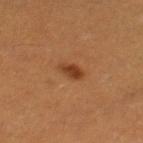<tbp_lesion>
<biopsy_status>not biopsied; imaged during a skin examination</biopsy_status>
<lesion_size>
  <long_diameter_mm_approx>3.0</long_diameter_mm_approx>
</lesion_size>
<image>
  <source>total-body photography crop</source>
  <field_of_view_mm>15</field_of_view_mm>
</image>
<site>leg</site>
<patient>
  <sex>female</sex>
  <age_approx>30</age_approx>
</patient>
</tbp_lesion>